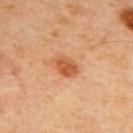<tbp_lesion>
  <biopsy_status>not biopsied; imaged during a skin examination</biopsy_status>
  <patient>
    <sex>male</sex>
    <age_approx>45</age_approx>
  </patient>
  <lesion_size>
    <long_diameter_mm_approx>3.0</long_diameter_mm_approx>
  </lesion_size>
  <site>back</site>
  <lighting>cross-polarized</lighting>
  <image>
    <source>total-body photography crop</source>
    <field_of_view_mm>15</field_of_view_mm>
  </image>
</tbp_lesion>